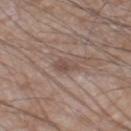<lesion>
  <biopsy_status>not biopsied; imaged during a skin examination</biopsy_status>
  <site>leg</site>
  <image>
    <source>total-body photography crop</source>
    <field_of_view_mm>15</field_of_view_mm>
  </image>
  <automated_metrics>
    <area_mm2_approx>4.0</area_mm2_approx>
    <eccentricity>0.75</eccentricity>
    <shape_asymmetry>0.3</shape_asymmetry>
    <cielab_L>49</cielab_L>
    <cielab_a>15</cielab_a>
    <cielab_b>23</cielab_b>
    <vs_skin_contrast_norm>6.0</vs_skin_contrast_norm>
    <nevus_likeness_0_100>0</nevus_likeness_0_100>
    <lesion_detection_confidence_0_100>95</lesion_detection_confidence_0_100>
  </automated_metrics>
  <lighting>white-light</lighting>
  <patient>
    <sex>male</sex>
    <age_approx>60</age_approx>
  </patient>
</lesion>